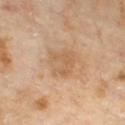<case>
<biopsy_status>not biopsied; imaged during a skin examination</biopsy_status>
<site>chest</site>
<image>
  <source>total-body photography crop</source>
  <field_of_view_mm>15</field_of_view_mm>
</image>
<patient>
  <sex>male</sex>
  <age_approx>70</age_approx>
</patient>
<lighting>cross-polarized</lighting>
<lesion_size>
  <long_diameter_mm_approx>4.0</long_diameter_mm_approx>
</lesion_size>
</case>